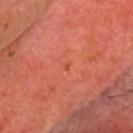Assessment:
This lesion was catalogued during total-body skin photography and was not selected for biopsy.
Background:
A lesion tile, about 15 mm wide, cut from a 3D total-body photograph. A male subject, about 70 years old. Measured at roughly 1 mm in maximum diameter. This is a cross-polarized tile. The lesion is on the head or neck.